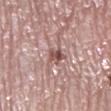Clinical summary: A female subject aged 63–67. Approximately 2.5 mm at its widest. A 15 mm close-up tile from a total-body photography series done for melanoma screening. From the left lower leg.